The lesion is on the back; this image is a 15 mm lesion crop taken from a total-body photograph; the patient is a male in their mid- to late 60s:
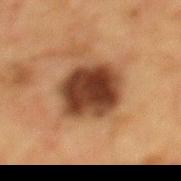<case>
  <lesion_size>
    <long_diameter_mm_approx>5.5</long_diameter_mm_approx>
  </lesion_size>
  <lighting>cross-polarized</lighting>
  <diagnosis>
    <histopathology>melanocytic nevus</histopathology>
    <malignancy>benign</malignancy>
    <taxonomic_path>Benign, Benign melanocytic proliferations, Nevus</taxonomic_path>
  </diagnosis>
</case>